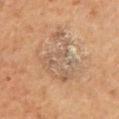Captured during whole-body skin photography for melanoma surveillance; the lesion was not biopsied. The total-body-photography lesion software estimated an area of roughly 21 mm² and an outline eccentricity of about 0.75 (0 = round, 1 = elongated). And it measured a border-irregularity rating of about 9/10, a within-lesion color-variation index near 4.5/10, and peripheral color asymmetry of about 1.5. The analysis additionally found an automated nevus-likeness rating near 0 out of 100 and lesion-presence confidence of about 100/100. From the lower back. A male subject, aged 63 to 67. A region of skin cropped from a whole-body photographic capture, roughly 15 mm wide. About 7.5 mm across.